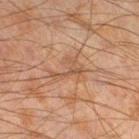Findings:
- notes — imaged on a skin check; not biopsied
- image — total-body-photography crop, ~15 mm field of view
- subject — male, roughly 45 years of age
- site — the left lower leg
- tile lighting — cross-polarized